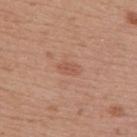Clinical impression:
Part of a total-body skin-imaging series; this lesion was reviewed on a skin check and was not flagged for biopsy.
Clinical summary:
A female subject, aged 38 to 42. Automated tile analysis of the lesion measured an average lesion color of about L≈55 a*≈23 b*≈31 (CIELAB) and a normalized lesion–skin contrast near 5. The lesion's longest dimension is about 3 mm. A roughly 15 mm field-of-view crop from a total-body skin photograph. Imaged with white-light lighting. From the upper back.